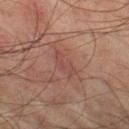workup = catalogued during a skin exam; not biopsied | lighting = cross-polarized | lesion diameter = ~4 mm (longest diameter) | body site = the leg | acquisition = 15 mm crop, total-body photography | patient = male, aged 73–77.